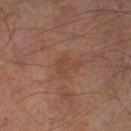{
  "lighting": "cross-polarized",
  "patient": {
    "sex": "male",
    "age_approx": 65
  },
  "image": {
    "source": "total-body photography crop",
    "field_of_view_mm": 15
  },
  "site": "leg",
  "lesion_size": {
    "long_diameter_mm_approx": 2.5
  }
}